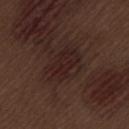workup: imaged on a skin check; not biopsied | automated lesion analysis: an average lesion color of about L≈21 a*≈17 b*≈17 (CIELAB), roughly 5 lightness units darker than nearby skin, and a normalized border contrast of about 6.5; a border-irregularity index near 3/10 and a color-variation rating of about 3.5/10; lesion-presence confidence of about 95/100 | patient: male, aged around 70 | tile lighting: white-light | site: the lower back | acquisition: ~15 mm tile from a whole-body skin photo | size: ~5 mm (longest diameter).